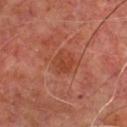notes: imaged on a skin check; not biopsied
site: the chest
image: 15 mm crop, total-body photography
patient: male, roughly 60 years of age